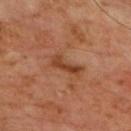{
  "biopsy_status": "not biopsied; imaged during a skin examination",
  "lighting": "cross-polarized",
  "site": "chest",
  "automated_metrics": {
    "area_mm2_approx": 5.0,
    "border_irregularity_0_10": 4.5,
    "color_variation_0_10": 3.0,
    "nevus_likeness_0_100": 0
  },
  "image": {
    "source": "total-body photography crop",
    "field_of_view_mm": 15
  },
  "lesion_size": {
    "long_diameter_mm_approx": 4.0
  },
  "patient": {
    "sex": "male",
    "age_approx": 60
  }
}